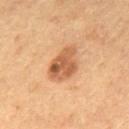Part of a total-body skin-imaging series; this lesion was reviewed on a skin check and was not flagged for biopsy.
A male patient approximately 55 years of age.
The lesion is on the mid back.
A roughly 15 mm field-of-view crop from a total-body skin photograph.
Imaged with cross-polarized lighting.
The lesion's longest dimension is about 5 mm.